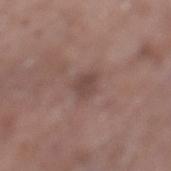{
  "biopsy_status": "not biopsied; imaged during a skin examination",
  "image": {
    "source": "total-body photography crop",
    "field_of_view_mm": 15
  },
  "patient": {
    "sex": "female",
    "age_approx": "85+"
  },
  "automated_metrics": {
    "area_mm2_approx": 4.5,
    "eccentricity": 0.5,
    "shape_asymmetry": 0.25,
    "nevus_likeness_0_100": 0
  },
  "lesion_size": {
    "long_diameter_mm_approx": 2.5
  },
  "lighting": "white-light",
  "site": "leg"
}